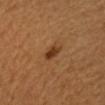{
  "biopsy_status": "not biopsied; imaged during a skin examination",
  "lighting": "cross-polarized",
  "site": "arm",
  "patient": {
    "sex": "female",
    "age_approx": 45
  },
  "automated_metrics": {
    "area_mm2_approx": 4.0,
    "shape_asymmetry": 0.3,
    "nevus_likeness_0_100": 95,
    "lesion_detection_confidence_0_100": 100
  },
  "image": {
    "source": "total-body photography crop",
    "field_of_view_mm": 15
  }
}